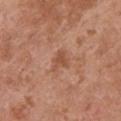Part of a total-body skin-imaging series; this lesion was reviewed on a skin check and was not flagged for biopsy. From the chest. Captured under white-light illumination. The subject is a female in their mid-60s. A 15 mm close-up tile from a total-body photography series done for melanoma screening.